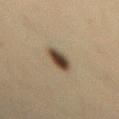Case summary:
* notes: total-body-photography surveillance lesion; no biopsy
* diameter: about 3 mm
* lighting: cross-polarized illumination
* subject: female, aged approximately 45
* automated lesion analysis: a footprint of about 6 mm² and a shape eccentricity near 0.7; a lesion color around L≈37 a*≈11 b*≈24 in CIELAB, a lesion–skin lightness drop of about 15, and a lesion-to-skin contrast of about 12.5 (normalized; higher = more distinct)
* site: the mid back
* image source: 15 mm crop, total-body photography